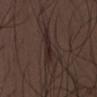| key | value |
|---|---|
| workup | catalogued during a skin exam; not biopsied |
| illumination | white-light illumination |
| subject | male, aged 28–32 |
| image source | ~15 mm tile from a whole-body skin photo |
| location | the abdomen |